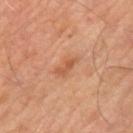The lesion was photographed on a routine skin check and not biopsied; there is no pathology result. A 15 mm crop from a total-body photograph taken for skin-cancer surveillance. Automated tile analysis of the lesion measured a within-lesion color-variation index near 2/10. The lesion is located on the left upper arm. A male subject in their mid- to late 60s. The tile uses cross-polarized illumination. The recorded lesion diameter is about 3 mm.